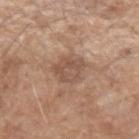The lesion was tiled from a total-body skin photograph and was not biopsied. A male patient, aged approximately 60. On the left forearm. A close-up tile cropped from a whole-body skin photograph, about 15 mm across.